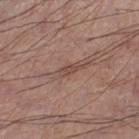{"biopsy_status": "not biopsied; imaged during a skin examination", "lighting": "white-light", "site": "right lower leg", "image": {"source": "total-body photography crop", "field_of_view_mm": 15}, "patient": {"sex": "male", "age_approx": 70}, "lesion_size": {"long_diameter_mm_approx": 3.0}, "automated_metrics": {"eccentricity": 0.9, "cielab_L": 47, "cielab_a": 18, "cielab_b": 25, "vs_skin_darker_L": 8.0}}